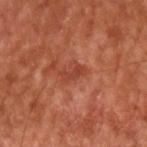<lesion>
<biopsy_status>not biopsied; imaged during a skin examination</biopsy_status>
<patient>
  <age_approx>65</age_approx>
</patient>
<site>arm</site>
<lesion_size>
  <long_diameter_mm_approx>2.5</long_diameter_mm_approx>
</lesion_size>
<image>
  <source>total-body photography crop</source>
  <field_of_view_mm>15</field_of_view_mm>
</image>
</lesion>